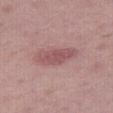| field | value |
|---|---|
| workup | total-body-photography surveillance lesion; no biopsy |
| patient | male, aged approximately 40 |
| site | the right thigh |
| lesion size | about 4.5 mm |
| illumination | white-light |
| imaging modality | total-body-photography crop, ~15 mm field of view |